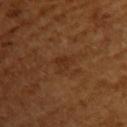Captured during whole-body skin photography for melanoma surveillance; the lesion was not biopsied.
Cropped from a total-body skin-imaging series; the visible field is about 15 mm.
Located on the left upper arm.
The patient is a female roughly 55 years of age.